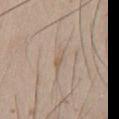Imaged during a routine full-body skin examination; the lesion was not biopsied and no histopathology is available.
Automated tile analysis of the lesion measured a lesion area of about 3 mm², an outline eccentricity of about 0.9 (0 = round, 1 = elongated), and two-axis asymmetry of about 0.25. It also reported a lesion color around L≈59 a*≈13 b*≈28 in CIELAB and a normalized border contrast of about 5.
A male patient, roughly 45 years of age.
From the chest.
Longest diameter approximately 3 mm.
Imaged with white-light lighting.
A 15 mm close-up extracted from a 3D total-body photography capture.